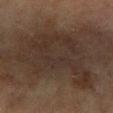Impression:
Imaged during a routine full-body skin examination; the lesion was not biopsied and no histopathology is available.
Background:
The lesion's longest dimension is about 10 mm. A female patient, approximately 80 years of age. On the left forearm. This image is a 15 mm lesion crop taken from a total-body photograph. The tile uses cross-polarized illumination.